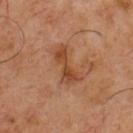imaging modality: ~15 mm tile from a whole-body skin photo
image-analysis metrics: a footprint of about 8.5 mm²; an average lesion color of about L≈46 a*≈24 b*≈35 (CIELAB), a lesion–skin lightness drop of about 9, and a lesion-to-skin contrast of about 7.5 (normalized; higher = more distinct); a border-irregularity index near 5/10, internal color variation of about 4.5 on a 0–10 scale, and radial color variation of about 1.5
location: the chest
patient: male, roughly 50 years of age
lesion diameter: about 5 mm
illumination: cross-polarized illumination